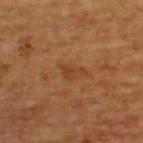Part of a total-body skin-imaging series; this lesion was reviewed on a skin check and was not flagged for biopsy. The lesion is on the upper back. Automated image analysis of the tile measured a footprint of about 3 mm² and a shape-asymmetry score of about 0.65 (0 = symmetric). And it measured a lesion color around L≈40 a*≈22 b*≈36 in CIELAB. It also reported a border-irregularity index near 7/10 and a peripheral color-asymmetry measure near 0.5. A male patient in their mid-60s. A close-up tile cropped from a whole-body skin photograph, about 15 mm across.